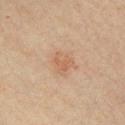Recorded during total-body skin imaging; not selected for excision or biopsy. Captured under cross-polarized illumination. A male subject aged approximately 35. Approximately 3 mm at its widest. An algorithmic analysis of the crop reported a footprint of about 5.5 mm², a shape eccentricity near 0.4, and two-axis asymmetry of about 0.3. And it measured a lesion–skin lightness drop of about 6. From the chest. A region of skin cropped from a whole-body photographic capture, roughly 15 mm wide.